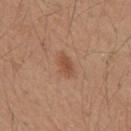Impression: Imaged during a routine full-body skin examination; the lesion was not biopsied and no histopathology is available. Image and clinical context: A 15 mm close-up tile from a total-body photography series done for melanoma screening. Imaged with white-light lighting. Located on the back. The subject is a male roughly 20 years of age. The recorded lesion diameter is about 3 mm.